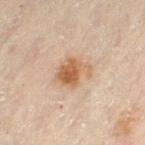{
  "biopsy_status": "not biopsied; imaged during a skin examination",
  "site": "leg",
  "lighting": "cross-polarized",
  "image": {
    "source": "total-body photography crop",
    "field_of_view_mm": 15
  },
  "lesion_size": {
    "long_diameter_mm_approx": 4.5
  },
  "patient": {
    "sex": "female",
    "age_approx": 60
  }
}